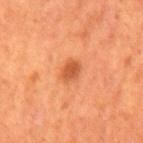Notes:
– biopsy status: catalogued during a skin exam; not biopsied
– subject: male, in their mid- to late 60s
– imaging modality: total-body-photography crop, ~15 mm field of view
– automated metrics: a lesion color around L≈51 a*≈29 b*≈39 in CIELAB, a lesion–skin lightness drop of about 10, and a normalized border contrast of about 7.5
– site: the mid back
– tile lighting: cross-polarized
– size: ~3 mm (longest diameter)